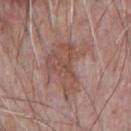Part of a total-body skin-imaging series; this lesion was reviewed on a skin check and was not flagged for biopsy.
The subject is a male roughly 70 years of age.
Automated tile analysis of the lesion measured a lesion area of about 20 mm², an outline eccentricity of about 0.75 (0 = round, 1 = elongated), and a shape-asymmetry score of about 0.55 (0 = symmetric). The analysis additionally found border irregularity of about 7.5 on a 0–10 scale, a within-lesion color-variation index near 4/10, and a peripheral color-asymmetry measure near 1.5.
The tile uses white-light illumination.
Located on the chest.
A close-up tile cropped from a whole-body skin photograph, about 15 mm across.
The recorded lesion diameter is about 7 mm.